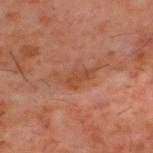Captured during whole-body skin photography for melanoma surveillance; the lesion was not biopsied. A male patient, roughly 60 years of age. From the back. A roughly 15 mm field-of-view crop from a total-body skin photograph.